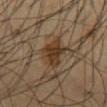workup: total-body-photography surveillance lesion; no biopsy | acquisition: ~15 mm tile from a whole-body skin photo | body site: the abdomen | lesion diameter: about 4 mm | tile lighting: cross-polarized | subject: male, aged approximately 60.